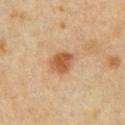Recorded during total-body skin imaging; not selected for excision or biopsy. A male subject, in their mid- to late 50s. A 15 mm crop from a total-body photograph taken for skin-cancer surveillance. Located on the arm. Measured at roughly 3 mm in maximum diameter.